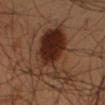{
  "biopsy_status": "not biopsied; imaged during a skin examination",
  "patient": {
    "sex": "male",
    "age_approx": 55
  },
  "site": "right forearm",
  "image": {
    "source": "total-body photography crop",
    "field_of_view_mm": 15
  },
  "lesion_size": {
    "long_diameter_mm_approx": 9.0
  }
}